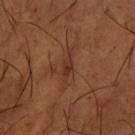This lesion was catalogued during total-body skin photography and was not selected for biopsy. A male subject, roughly 65 years of age. Longest diameter approximately 4.5 mm. The tile uses cross-polarized illumination. The lesion is located on the arm. A region of skin cropped from a whole-body photographic capture, roughly 15 mm wide.